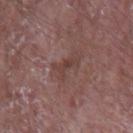On the arm. Approximately 3.5 mm at its widest. A lesion tile, about 15 mm wide, cut from a 3D total-body photograph. Automated image analysis of the tile measured an area of roughly 4.5 mm² and a shape eccentricity near 0.9. And it measured a mean CIELAB color near L≈40 a*≈19 b*≈21 and a normalized border contrast of about 6. The analysis additionally found border irregularity of about 5.5 on a 0–10 scale, a color-variation rating of about 0/10, and radial color variation of about 0. A male patient, roughly 65 years of age. Captured under white-light illumination.